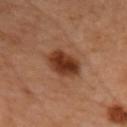This lesion was catalogued during total-body skin photography and was not selected for biopsy. A female patient aged 58 to 62. The lesion is located on the head or neck. A lesion tile, about 15 mm wide, cut from a 3D total-body photograph.